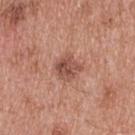Case summary:
* biopsy status: total-body-photography surveillance lesion; no biopsy